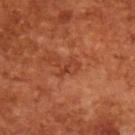Findings:
• workup — imaged on a skin check; not biopsied
• tile lighting — cross-polarized
• patient — male, in their mid-60s
• automated lesion analysis — a border-irregularity rating of about 5/10 and internal color variation of about 0 on a 0–10 scale; an automated nevus-likeness rating near 0 out of 100
• imaging modality — total-body-photography crop, ~15 mm field of view